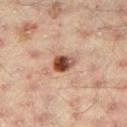Q: Was this lesion biopsied?
A: catalogued during a skin exam; not biopsied
Q: Lesion size?
A: ≈2.5 mm
Q: Who is the patient?
A: male, aged approximately 45
Q: What is the imaging modality?
A: 15 mm crop, total-body photography
Q: What is the anatomic site?
A: the right thigh
Q: Illumination type?
A: cross-polarized illumination